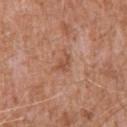Notes:
– workup: imaged on a skin check; not biopsied
– acquisition: ~15 mm crop, total-body skin-cancer survey
– lighting: white-light illumination
– automated metrics: an area of roughly 3 mm², an eccentricity of roughly 0.8, and two-axis asymmetry of about 0.45; border irregularity of about 5 on a 0–10 scale and a color-variation rating of about 1/10; a classifier nevus-likeness of about 0/100 and lesion-presence confidence of about 100/100
– lesion size: about 2.5 mm
– body site: the upper back
– patient: male, approximately 60 years of age